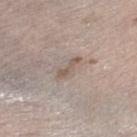Notes:
• biopsy status · imaged on a skin check; not biopsied
• image source · total-body-photography crop, ~15 mm field of view
• patient · male, aged 68 to 72
• anatomic site · the right lower leg